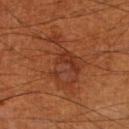Assessment:
Captured during whole-body skin photography for melanoma surveillance; the lesion was not biopsied.
Clinical summary:
A male subject aged 68 to 72. A 15 mm close-up tile from a total-body photography series done for melanoma screening. The lesion is on the right thigh. Approximately 7 mm at its widest. The tile uses cross-polarized illumination.